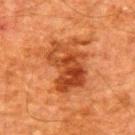No biopsy was performed on this lesion — it was imaged during a full skin examination and was not determined to be concerning. The total-body-photography lesion software estimated a lesion area of about 19 mm² and two-axis asymmetry of about 0.3. The software also gave a lesion color around L≈37 a*≈27 b*≈35 in CIELAB, a lesion–skin lightness drop of about 10, and a normalized border contrast of about 8.5. The software also gave an automated nevus-likeness rating near 70 out of 100 and a lesion-detection confidence of about 100/100. From the back. A roughly 15 mm field-of-view crop from a total-body skin photograph. A male patient, aged around 60. Approximately 6 mm at its widest. Imaged with cross-polarized lighting.